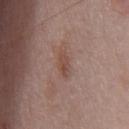The lesion was photographed on a routine skin check and not biopsied; there is no pathology result. The tile uses white-light illumination. A male patient aged 53–57. A 15 mm close-up tile from a total-body photography series done for melanoma screening. Located on the mid back.